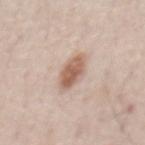notes = catalogued during a skin exam; not biopsied.